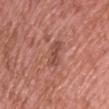Q: Was a biopsy performed?
A: no biopsy performed (imaged during a skin exam)
Q: What kind of image is this?
A: 15 mm crop, total-body photography
Q: Automated lesion metrics?
A: an area of roughly 5 mm² and a symmetry-axis asymmetry near 0.35; a classifier nevus-likeness of about 0/100
Q: Where on the body is the lesion?
A: the upper back
Q: What is the lesion's diameter?
A: ≈3.5 mm
Q: What lighting was used for the tile?
A: white-light illumination
Q: Who is the patient?
A: male, in their 70s